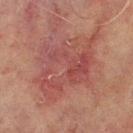biopsy status=catalogued during a skin exam; not biopsied
subject=male, aged around 70
illumination=cross-polarized illumination
lesion size=about 9 mm
location=the chest
acquisition=~15 mm tile from a whole-body skin photo